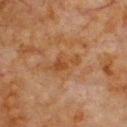Part of a total-body skin-imaging series; this lesion was reviewed on a skin check and was not flagged for biopsy.
This is a cross-polarized tile.
The lesion is located on the front of the torso.
Automated image analysis of the tile measured an automated nevus-likeness rating near 0 out of 100 and a detector confidence of about 100 out of 100 that the crop contains a lesion.
A region of skin cropped from a whole-body photographic capture, roughly 15 mm wide.
A male patient about 80 years old.
The recorded lesion diameter is about 4 mm.